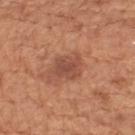Impression:
Imaged during a routine full-body skin examination; the lesion was not biopsied and no histopathology is available.
Background:
The lesion is on the mid back. A 15 mm close-up tile from a total-body photography series done for melanoma screening. About 3.5 mm across. Imaged with white-light lighting. A male subject aged around 65.